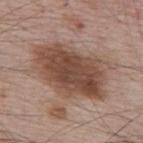{
  "biopsy_status": "not biopsied; imaged during a skin examination",
  "automated_metrics": {
    "area_mm2_approx": 36.0,
    "eccentricity": 0.8,
    "shape_asymmetry": 0.25,
    "nevus_likeness_0_100": 80
  },
  "site": "upper back",
  "patient": {
    "sex": "male",
    "age_approx": 65
  },
  "lesion_size": {
    "long_diameter_mm_approx": 9.0
  },
  "image": {
    "source": "total-body photography crop",
    "field_of_view_mm": 15
  },
  "lighting": "white-light"
}